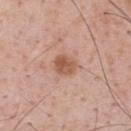Q: Lesion size?
A: about 3 mm
Q: What is the imaging modality?
A: total-body-photography crop, ~15 mm field of view
Q: What is the anatomic site?
A: the back
Q: What lighting was used for the tile?
A: white-light illumination
Q: Patient demographics?
A: male, aged 53–57